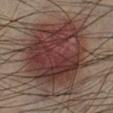biopsy status: no biopsy performed (imaged during a skin exam); image source: total-body-photography crop, ~15 mm field of view; size: ~11 mm (longest diameter); anatomic site: the left lower leg; patient: male, aged 43–47.